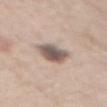<lesion>
  <biopsy_status>not biopsied; imaged during a skin examination</biopsy_status>
  <automated_metrics>
    <area_mm2_approx>9.5</area_mm2_approx>
    <eccentricity>0.8</eccentricity>
  </automated_metrics>
  <site>front of the torso</site>
  <patient>
    <sex>male</sex>
    <age_approx>60</age_approx>
  </patient>
  <lesion_size>
    <long_diameter_mm_approx>4.5</long_diameter_mm_approx>
  </lesion_size>
  <lighting>white-light</lighting>
  <image>
    <source>total-body photography crop</source>
    <field_of_view_mm>15</field_of_view_mm>
  </image>
</lesion>